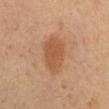  site: mid back
  lesion_size:
    long_diameter_mm_approx: 5.5
  image:
    source: total-body photography crop
    field_of_view_mm: 15
  lighting: cross-polarized
  patient:
    sex: male
    age_approx: 60